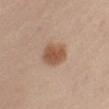| feature | finding |
|---|---|
| location | the arm |
| imaging modality | 15 mm crop, total-body photography |
| patient | male, aged 73–77 |